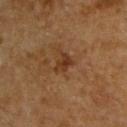Clinical summary:
The patient is a male aged 63 to 67. Imaged with cross-polarized lighting. From the back. A 15 mm crop from a total-body photograph taken for skin-cancer surveillance. The total-body-photography lesion software estimated an average lesion color of about L≈31 a*≈19 b*≈30 (CIELAB), roughly 8 lightness units darker than nearby skin, and a normalized lesion–skin contrast near 7.5. The analysis additionally found a border-irregularity index near 3.5/10, internal color variation of about 3 on a 0–10 scale, and peripheral color asymmetry of about 1. The software also gave a nevus-likeness score of about 5/100 and lesion-presence confidence of about 100/100. Measured at roughly 2.5 mm in maximum diameter.